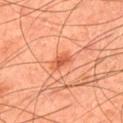Part of a total-body skin-imaging series; this lesion was reviewed on a skin check and was not flagged for biopsy.
Automated image analysis of the tile measured an average lesion color of about L≈56 a*≈33 b*≈40 (CIELAB), roughly 10 lightness units darker than nearby skin, and a normalized lesion–skin contrast near 7. The analysis additionally found a border-irregularity rating of about 3/10, a within-lesion color-variation index near 3/10, and peripheral color asymmetry of about 1.
A 15 mm close-up tile from a total-body photography series done for melanoma screening.
About 2.5 mm across.
The patient is a male roughly 45 years of age.
From the upper back.
Imaged with cross-polarized lighting.